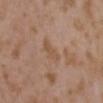Recorded during total-body skin imaging; not selected for excision or biopsy.
A region of skin cropped from a whole-body photographic capture, roughly 15 mm wide.
Measured at roughly 3.5 mm in maximum diameter.
Imaged with white-light lighting.
The patient is a female approximately 35 years of age.
The lesion is on the chest.